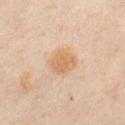Case summary:
• biopsy status · total-body-photography surveillance lesion; no biopsy
• image-analysis metrics · a footprint of about 6.5 mm², an outline eccentricity of about 0.6 (0 = round, 1 = elongated), and a shape-asymmetry score of about 0.2 (0 = symmetric); an average lesion color of about L≈64 a*≈19 b*≈36 (CIELAB), a lesion–skin lightness drop of about 8, and a normalized lesion–skin contrast near 7
• tile lighting · cross-polarized illumination
• image source · 15 mm crop, total-body photography
• size · about 3 mm
• site · the chest
• subject · female, in their mid-40s